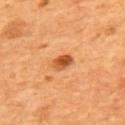{
  "biopsy_status": "not biopsied; imaged during a skin examination",
  "site": "back",
  "lighting": "cross-polarized",
  "patient": {
    "sex": "male",
    "age_approx": 55
  },
  "automated_metrics": {
    "cielab_L": 50,
    "cielab_a": 27,
    "cielab_b": 42,
    "vs_skin_darker_L": 13.0,
    "vs_skin_contrast_norm": 9.0,
    "peripheral_color_asymmetry": 2.0,
    "nevus_likeness_0_100": 90,
    "lesion_detection_confidence_0_100": 100
  },
  "image": {
    "source": "total-body photography crop",
    "field_of_view_mm": 15
  }
}